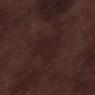Q: Was a biopsy performed?
A: no biopsy performed (imaged during a skin exam)
Q: Automated lesion metrics?
A: an automated nevus-likeness rating near 0 out of 100
Q: What is the anatomic site?
A: the left lower leg
Q: How was this image acquired?
A: total-body-photography crop, ~15 mm field of view
Q: Patient demographics?
A: male, aged 68 to 72
Q: Lesion size?
A: about 5 mm